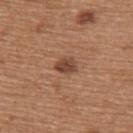workup = no biopsy performed (imaged during a skin exam); lighting = white-light; acquisition = 15 mm crop, total-body photography; patient = female, approximately 60 years of age; body site = the back.